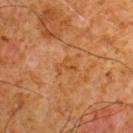Impression:
Part of a total-body skin-imaging series; this lesion was reviewed on a skin check and was not flagged for biopsy.
Background:
Approximately 2.5 mm at its widest. A male patient, aged 58–62. Cropped from a total-body skin-imaging series; the visible field is about 15 mm. The lesion is on the upper back.